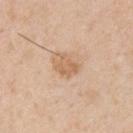This lesion was catalogued during total-body skin photography and was not selected for biopsy. On the upper back. A male subject, aged approximately 30. The tile uses white-light illumination. A close-up tile cropped from a whole-body skin photograph, about 15 mm across.